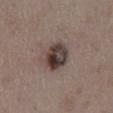Case summary:
– notes · imaged on a skin check; not biopsied
– lesion size · ≈4 mm
– location · the abdomen
– patient · male, approximately 75 years of age
– tile lighting · white-light
– image · 15 mm crop, total-body photography